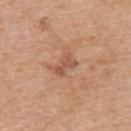lesion_size:
  long_diameter_mm_approx: 3.0
image:
  source: total-body photography crop
  field_of_view_mm: 15
patient:
  sex: male
  age_approx: 65
site: upper back
automated_metrics:
  border_irregularity_0_10: 5.0
  color_variation_0_10: 0.0
lighting: white-light